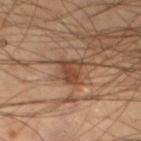The lesion was tiled from a total-body skin photograph and was not biopsied. Located on the left lower leg. The total-body-photography lesion software estimated a lesion area of about 5 mm², an outline eccentricity of about 0.5 (0 = round, 1 = elongated), and a shape-asymmetry score of about 0.3 (0 = symmetric). And it measured a within-lesion color-variation index near 3/10 and peripheral color asymmetry of about 1. The software also gave a detector confidence of about 100 out of 100 that the crop contains a lesion. Imaged with cross-polarized lighting. A lesion tile, about 15 mm wide, cut from a 3D total-body photograph. The subject is a male in their mid-40s. About 2.5 mm across.